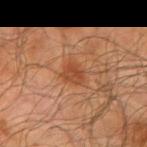notes = no biopsy performed (imaged during a skin exam).